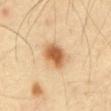| field | value |
|---|---|
| biopsy status | total-body-photography surveillance lesion; no biopsy |
| imaging modality | 15 mm crop, total-body photography |
| patient | male, about 40 years old |
| location | the abdomen |
| lesion diameter | about 4 mm |
| TBP lesion metrics | a lesion color around L≈62 a*≈21 b*≈40 in CIELAB, roughly 15 lightness units darker than nearby skin, and a normalized border contrast of about 9.5; a border-irregularity index near 1/10, a color-variation rating of about 5.5/10, and radial color variation of about 1.5 |
| illumination | cross-polarized |